Captured under white-light illumination; a lesion tile, about 15 mm wide, cut from a 3D total-body photograph; from the head or neck; measured at roughly 8.5 mm in maximum diameter; An algorithmic analysis of the crop reported an average lesion color of about L≈48 a*≈17 b*≈22 (CIELAB), about 8 CIELAB-L* units darker than the surrounding skin, and a normalized border contrast of about 6.5; the subject is a male aged 48 to 52.
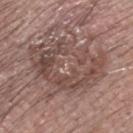biopsy diagnosis — a seborrheic keratosis.